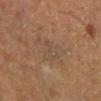Impression: Captured during whole-body skin photography for melanoma surveillance; the lesion was not biopsied. Context: The lesion is located on the leg. The lesion's longest dimension is about 5 mm. A male patient, in their 70s. A lesion tile, about 15 mm wide, cut from a 3D total-body photograph. Captured under cross-polarized illumination.